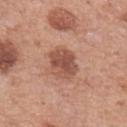This lesion was catalogued during total-body skin photography and was not selected for biopsy. The tile uses white-light illumination. The lesion-visualizer software estimated border irregularity of about 2 on a 0–10 scale, a color-variation rating of about 4.5/10, and peripheral color asymmetry of about 1.5. And it measured a classifier nevus-likeness of about 55/100 and a detector confidence of about 100 out of 100 that the crop contains a lesion. A 15 mm close-up tile from a total-body photography series done for melanoma screening. Longest diameter approximately 4 mm. The lesion is on the left forearm. The patient is a female approximately 60 years of age.